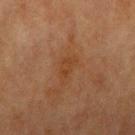Q: Was a biopsy performed?
A: no biopsy performed (imaged during a skin exam)
Q: What lighting was used for the tile?
A: cross-polarized
Q: Patient demographics?
A: female, in their mid- to late 50s
Q: Lesion location?
A: the right upper arm
Q: Lesion size?
A: ≈2.5 mm
Q: How was this image acquired?
A: ~15 mm crop, total-body skin-cancer survey